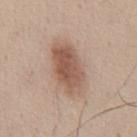Assessment: This lesion was catalogued during total-body skin photography and was not selected for biopsy. Clinical summary: From the abdomen. The total-body-photography lesion software estimated a lesion area of about 17 mm² and an eccentricity of roughly 0.8. And it measured a classifier nevus-likeness of about 95/100 and a detector confidence of about 100 out of 100 that the crop contains a lesion. This image is a 15 mm lesion crop taken from a total-body photograph. Captured under white-light illumination. About 6 mm across. A male subject, aged 43 to 47.